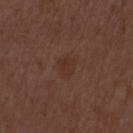Part of a total-body skin-imaging series; this lesion was reviewed on a skin check and was not flagged for biopsy. The patient is a male aged 48 to 52. This image is a 15 mm lesion crop taken from a total-body photograph.